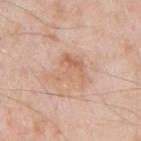Clinical impression:
This lesion was catalogued during total-body skin photography and was not selected for biopsy.
Acquisition and patient details:
The lesion is located on the left upper arm. Cropped from a whole-body photographic skin survey; the tile spans about 15 mm. The subject is a male aged 53–57.